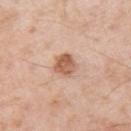Captured during whole-body skin photography for melanoma surveillance; the lesion was not biopsied.
A region of skin cropped from a whole-body photographic capture, roughly 15 mm wide.
Located on the left upper arm.
The patient is a male about 55 years old.
The tile uses white-light illumination.
About 3 mm across.